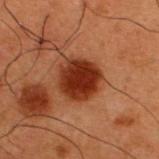Recorded during total-body skin imaging; not selected for excision or biopsy.
Automated tile analysis of the lesion measured a footprint of about 14 mm² and a shape eccentricity near 0.4. The analysis additionally found a nevus-likeness score of about 100/100 and lesion-presence confidence of about 100/100.
The patient is a male aged approximately 50.
The lesion is on the upper back.
Captured under cross-polarized illumination.
A close-up tile cropped from a whole-body skin photograph, about 15 mm across.
Approximately 4.5 mm at its widest.